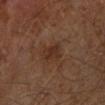| field | value |
|---|---|
| size | about 3 mm |
| automated lesion analysis | an area of roughly 5.5 mm², an outline eccentricity of about 0.7 (0 = round, 1 = elongated), and a shape-asymmetry score of about 0.25 (0 = symmetric); a border-irregularity index near 2/10, a color-variation rating of about 2.5/10, and radial color variation of about 1 |
| image | 15 mm crop, total-body photography |
| site | the leg |
| illumination | cross-polarized illumination |
| patient | male, approximately 60 years of age |